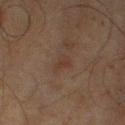image source=15 mm crop, total-body photography
site=the left lower leg
patient=male, roughly 45 years of age